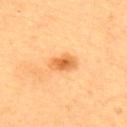On the upper back. The patient is a female aged 58–62. The tile uses cross-polarized illumination. A lesion tile, about 15 mm wide, cut from a 3D total-body photograph. About 3.5 mm across.